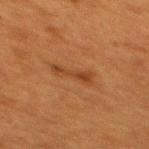Imaged during a routine full-body skin examination; the lesion was not biopsied and no histopathology is available.
The subject is a female roughly 40 years of age.
Cropped from a total-body skin-imaging series; the visible field is about 15 mm.
Automated tile analysis of the lesion measured a border-irregularity rating of about 4.5/10, internal color variation of about 1 on a 0–10 scale, and peripheral color asymmetry of about 0. The analysis additionally found a nevus-likeness score of about 0/100 and lesion-presence confidence of about 100/100.
Approximately 4.5 mm at its widest.
The lesion is on the upper back.